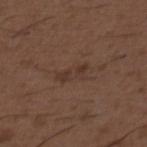workup: no biopsy performed (imaged during a skin exam); image source: ~15 mm crop, total-body skin-cancer survey; subject: male, in their 50s; body site: the back.